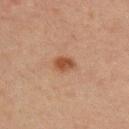From the upper back. A male subject roughly 30 years of age. The recorded lesion diameter is about 3 mm. Cropped from a total-body skin-imaging series; the visible field is about 15 mm.